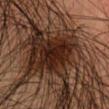| key | value |
|---|---|
| notes | no biopsy performed (imaged during a skin exam) |
| image | ~15 mm crop, total-body skin-cancer survey |
| body site | the head or neck |
| patient | male, in their mid- to late 30s |